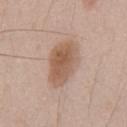No biopsy was performed on this lesion — it was imaged during a full skin examination and was not determined to be concerning. The tile uses white-light illumination. A male patient, aged 48–52. A roughly 15 mm field-of-view crop from a total-body skin photograph. About 6 mm across. The lesion is on the chest.